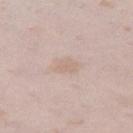{
  "biopsy_status": "not biopsied; imaged during a skin examination",
  "image": {
    "source": "total-body photography crop",
    "field_of_view_mm": 15
  },
  "lighting": "white-light",
  "site": "left thigh",
  "lesion_size": {
    "long_diameter_mm_approx": 2.5
  },
  "patient": {
    "sex": "female",
    "age_approx": 25
  }
}